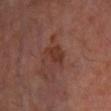Findings:
• follow-up: no biopsy performed (imaged during a skin exam)
• body site: the right lower leg
• image: 15 mm crop, total-body photography
• illumination: cross-polarized illumination
• subject: male, roughly 70 years of age